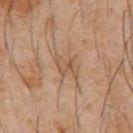follow-up: imaged on a skin check; not biopsied | imaging modality: ~15 mm crop, total-body skin-cancer survey | location: the chest | lighting: cross-polarized | subject: male, aged approximately 60 | diameter: ~3.5 mm (longest diameter).